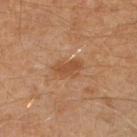Recorded during total-body skin imaging; not selected for excision or biopsy. On the left leg. Imaged with cross-polarized lighting. The lesion-visualizer software estimated a lesion area of about 5.5 mm², an eccentricity of roughly 0.8, and two-axis asymmetry of about 0.2. It also reported a border-irregularity rating of about 2/10, internal color variation of about 2 on a 0–10 scale, and peripheral color asymmetry of about 0.5. The software also gave a classifier nevus-likeness of about 25/100 and lesion-presence confidence of about 100/100. The subject is a male aged 28–32. Approximately 3.5 mm at its widest. Cropped from a whole-body photographic skin survey; the tile spans about 15 mm.